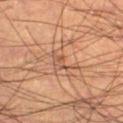Imaged during a routine full-body skin examination; the lesion was not biopsied and no histopathology is available.
A region of skin cropped from a whole-body photographic capture, roughly 15 mm wide.
Located on the right thigh.
Longest diameter approximately 3.5 mm.
A male subject aged 53–57.